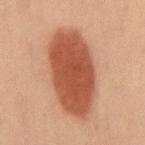follow-up: imaged on a skin check; not biopsied
automated lesion analysis: border irregularity of about 2 on a 0–10 scale, a within-lesion color-variation index near 2.5/10, and peripheral color asymmetry of about 1
tile lighting: cross-polarized illumination
body site: the mid back
imaging modality: total-body-photography crop, ~15 mm field of view
diameter: about 10 mm
subject: male, approximately 60 years of age